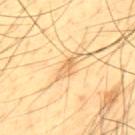Assessment:
Recorded during total-body skin imaging; not selected for excision or biopsy.
Acquisition and patient details:
Located on the mid back. A region of skin cropped from a whole-body photographic capture, roughly 15 mm wide. Measured at roughly 3.5 mm in maximum diameter. The tile uses cross-polarized illumination. A male patient, about 45 years old.